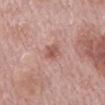Assessment:
The lesion was photographed on a routine skin check and not biopsied; there is no pathology result.
Clinical summary:
The recorded lesion diameter is about 2.5 mm. A close-up tile cropped from a whole-body skin photograph, about 15 mm across. On the mid back. Imaged with white-light lighting. A male subject, aged around 75. An algorithmic analysis of the crop reported a mean CIELAB color near L≈55 a*≈24 b*≈26, roughly 10 lightness units darker than nearby skin, and a lesion-to-skin contrast of about 7 (normalized; higher = more distinct). The software also gave a border-irregularity index near 3/10, internal color variation of about 2.5 on a 0–10 scale, and radial color variation of about 1. The software also gave a classifier nevus-likeness of about 35/100 and a detector confidence of about 100 out of 100 that the crop contains a lesion.